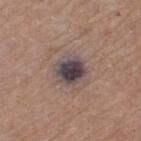Recorded during total-body skin imaging; not selected for excision or biopsy. A female subject, in their mid-70s. A 15 mm close-up tile from a total-body photography series done for melanoma screening. From the left thigh. The total-body-photography lesion software estimated a footprint of about 9.5 mm², an outline eccentricity of about 0.35 (0 = round, 1 = elongated), and two-axis asymmetry of about 0.1. The software also gave a lesion color around L≈42 a*≈12 b*≈12 in CIELAB, a lesion–skin lightness drop of about 14, and a normalized border contrast of about 13.5. And it measured a nevus-likeness score of about 60/100 and lesion-presence confidence of about 100/100. Captured under white-light illumination. Approximately 3.5 mm at its widest.